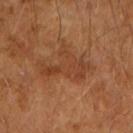The lesion was photographed on a routine skin check and not biopsied; there is no pathology result.
The tile uses cross-polarized illumination.
A male subject roughly 65 years of age.
Automated image analysis of the tile measured an average lesion color of about L≈41 a*≈23 b*≈33 (CIELAB), roughly 7 lightness units darker than nearby skin, and a normalized lesion–skin contrast near 5.5. The software also gave internal color variation of about 3.5 on a 0–10 scale and peripheral color asymmetry of about 1.
This image is a 15 mm lesion crop taken from a total-body photograph.
Measured at roughly 6.5 mm in maximum diameter.